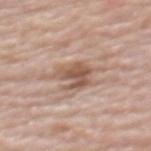The lesion was photographed on a routine skin check and not biopsied; there is no pathology result. A female patient about 65 years old. Located on the upper back. The tile uses white-light illumination. Cropped from a total-body skin-imaging series; the visible field is about 15 mm. Measured at roughly 4 mm in maximum diameter.